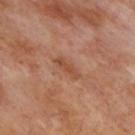Q: Was a biopsy performed?
A: imaged on a skin check; not biopsied
Q: Lesion location?
A: the upper back
Q: What kind of image is this?
A: total-body-photography crop, ~15 mm field of view
Q: How was the tile lit?
A: cross-polarized illumination
Q: Patient demographics?
A: male, aged approximately 70